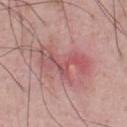workup = catalogued during a skin exam; not biopsied
illumination = white-light
lesion size = ~6.5 mm (longest diameter)
site = the front of the torso
imaging modality = 15 mm crop, total-body photography
patient = male, aged 48 to 52
automated lesion analysis = a shape eccentricity near 0.9 and two-axis asymmetry of about 0.6; about 9 CIELAB-L* units darker than the surrounding skin and a normalized border contrast of about 6; border irregularity of about 9.5 on a 0–10 scale, internal color variation of about 9 on a 0–10 scale, and radial color variation of about 4